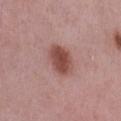Captured during whole-body skin photography for melanoma surveillance; the lesion was not biopsied. A 15 mm crop from a total-body photograph taken for skin-cancer surveillance. A female patient, about 40 years old. An algorithmic analysis of the crop reported a footprint of about 8.5 mm² and an outline eccentricity of about 0.6 (0 = round, 1 = elongated). Imaged with white-light lighting. The lesion is on the left thigh.